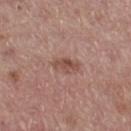The lesion was photographed on a routine skin check and not biopsied; there is no pathology result. From the left thigh. The lesion's longest dimension is about 2.5 mm. A male subject, approximately 55 years of age. A close-up tile cropped from a whole-body skin photograph, about 15 mm across.